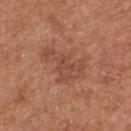Impression: No biopsy was performed on this lesion — it was imaged during a full skin examination and was not determined to be concerning. Acquisition and patient details: Cropped from a whole-body photographic skin survey; the tile spans about 15 mm. A female patient, approximately 40 years of age. The lesion is located on the upper back. The recorded lesion diameter is about 5.5 mm. The tile uses white-light illumination.